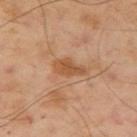Assessment:
Imaged during a routine full-body skin examination; the lesion was not biopsied and no histopathology is available.
Context:
Longest diameter approximately 3.5 mm. On the left arm. The patient is a male in their 50s. A region of skin cropped from a whole-body photographic capture, roughly 15 mm wide. This is a cross-polarized tile. Automated image analysis of the tile measured a normalized lesion–skin contrast near 7. The analysis additionally found a classifier nevus-likeness of about 0/100 and a lesion-detection confidence of about 100/100.